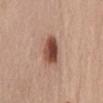Longest diameter approximately 4 mm. A female subject in their 50s. A 15 mm close-up tile from a total-body photography series done for melanoma screening. This is a white-light tile. From the abdomen.